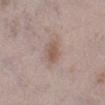Case summary:
- notes — no biopsy performed (imaged during a skin exam)
- patient — female, in their 60s
- site — the left lower leg
- automated lesion analysis — a lesion area of about 5.5 mm² and an eccentricity of roughly 0.6; a mean CIELAB color near L≈55 a*≈16 b*≈24, a lesion–skin lightness drop of about 8, and a normalized lesion–skin contrast near 6.5; a border-irregularity index near 1.5/10, a within-lesion color-variation index near 2.5/10, and peripheral color asymmetry of about 1; a classifier nevus-likeness of about 55/100 and lesion-presence confidence of about 100/100
- lighting — white-light illumination
- lesion size — ≈2.5 mm
- image — ~15 mm tile from a whole-body skin photo